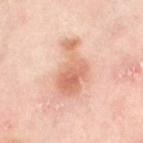Assessment:
This lesion was catalogued during total-body skin photography and was not selected for biopsy.